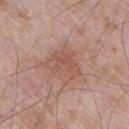{"biopsy_status": "not biopsied; imaged during a skin examination", "lesion_size": {"long_diameter_mm_approx": 5.0}, "patient": {"sex": "male", "age_approx": 70}, "lighting": "white-light", "site": "right thigh", "automated_metrics": {"eccentricity": 0.7, "shape_asymmetry": 0.35, "nevus_likeness_0_100": 5}, "image": {"source": "total-body photography crop", "field_of_view_mm": 15}}